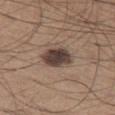Imaged during a routine full-body skin examination; the lesion was not biopsied and no histopathology is available.
A region of skin cropped from a whole-body photographic capture, roughly 15 mm wide.
This is a white-light tile.
A male subject, roughly 65 years of age.
Measured at roughly 3.5 mm in maximum diameter.
From the leg.